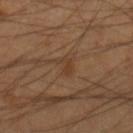follow-up — total-body-photography surveillance lesion; no biopsy.